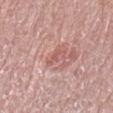| key | value |
|---|---|
| biopsy status | imaged on a skin check; not biopsied |
| TBP lesion metrics | an area of roughly 3.5 mm², an eccentricity of roughly 0.85, and two-axis asymmetry of about 0.45; an average lesion color of about L≈57 a*≈25 b*≈24 (CIELAB) and roughly 8 lightness units darker than nearby skin; a border-irregularity index near 6.5/10, a color-variation rating of about 0/10, and a peripheral color-asymmetry measure near 0; lesion-presence confidence of about 60/100 |
| body site | the left upper arm |
| lesion diameter | ≈3 mm |
| acquisition | ~15 mm crop, total-body skin-cancer survey |
| subject | female, aged around 65 |
| lighting | white-light |